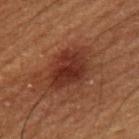Q: Was this lesion biopsied?
A: catalogued during a skin exam; not biopsied
Q: Who is the patient?
A: male, in their 50s
Q: What is the anatomic site?
A: the left upper arm
Q: What kind of image is this?
A: ~15 mm crop, total-body skin-cancer survey
Q: How large is the lesion?
A: about 5 mm